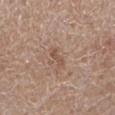Q: What is the anatomic site?
A: the left lower leg
Q: Who is the patient?
A: male, aged 63 to 67
Q: Illumination type?
A: white-light
Q: What kind of image is this?
A: ~15 mm crop, total-body skin-cancer survey
Q: What is the lesion's diameter?
A: about 2.5 mm